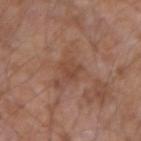Findings:
– follow-up · imaged on a skin check; not biopsied
– lighting · white-light illumination
– image source · 15 mm crop, total-body photography
– TBP lesion metrics · an average lesion color of about L≈45 a*≈21 b*≈29 (CIELAB), a lesion–skin lightness drop of about 6, and a normalized border contrast of about 5.5
– anatomic site · the left forearm
– patient · male, roughly 55 years of age
– size · ≈2.5 mm